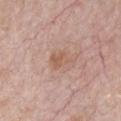The lesion was tiled from a total-body skin photograph and was not biopsied. The lesion-visualizer software estimated a footprint of about 4.5 mm², an outline eccentricity of about 0.7 (0 = round, 1 = elongated), and two-axis asymmetry of about 0.25. It also reported border irregularity of about 3 on a 0–10 scale. A region of skin cropped from a whole-body photographic capture, roughly 15 mm wide. Imaged with white-light lighting. The lesion is located on the chest. The subject is a female aged around 65.